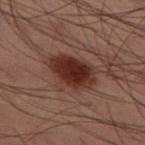Notes:
• size — ≈6 mm
• site — the leg
• automated metrics — an average lesion color of about L≈24 a*≈18 b*≈19 (CIELAB), about 10 CIELAB-L* units darker than the surrounding skin, and a lesion-to-skin contrast of about 11 (normalized; higher = more distinct); a border-irregularity rating of about 3/10 and peripheral color asymmetry of about 1
• subject — male, in their mid-50s
• image — 15 mm crop, total-body photography
• tile lighting — cross-polarized illumination